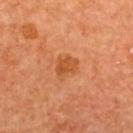Captured during whole-body skin photography for melanoma surveillance; the lesion was not biopsied. The patient is a female approximately 65 years of age. Approximately 3 mm at its widest. The tile uses cross-polarized illumination. A lesion tile, about 15 mm wide, cut from a 3D total-body photograph. An algorithmic analysis of the crop reported an area of roughly 6 mm², a shape eccentricity near 0.55, and a shape-asymmetry score of about 0.3 (0 = symmetric). The software also gave a within-lesion color-variation index near 2.5/10 and a peripheral color-asymmetry measure near 1. On the back.